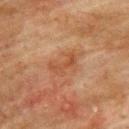Q: Is there a histopathology result?
A: catalogued during a skin exam; not biopsied
Q: What kind of image is this?
A: total-body-photography crop, ~15 mm field of view
Q: Who is the patient?
A: male, about 75 years old
Q: What is the anatomic site?
A: the upper back